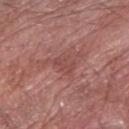Assessment: Part of a total-body skin-imaging series; this lesion was reviewed on a skin check and was not flagged for biopsy. Background: Imaged with white-light lighting. A male subject, about 60 years old. A 15 mm close-up tile from a total-body photography series done for melanoma screening. Located on the right forearm.